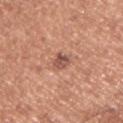Impression: Part of a total-body skin-imaging series; this lesion was reviewed on a skin check and was not flagged for biopsy. Context: Located on the right upper arm. Cropped from a total-body skin-imaging series; the visible field is about 15 mm. The patient is a male roughly 55 years of age.